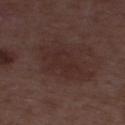– biopsy status · catalogued during a skin exam; not biopsied
– image source · ~15 mm crop, total-body skin-cancer survey
– diameter · about 7 mm
– tile lighting · white-light illumination
– patient · male, aged 48–52